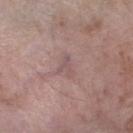Clinical impression: Part of a total-body skin-imaging series; this lesion was reviewed on a skin check and was not flagged for biopsy. Acquisition and patient details: Approximately 2.5 mm at its widest. A close-up tile cropped from a whole-body skin photograph, about 15 mm across. On the right lower leg. The total-body-photography lesion software estimated border irregularity of about 6 on a 0–10 scale, a color-variation rating of about 0/10, and a peripheral color-asymmetry measure near 0. A female patient approximately 75 years of age.